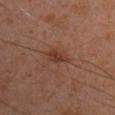Assessment: This lesion was catalogued during total-body skin photography and was not selected for biopsy. Context: Cropped from a whole-body photographic skin survey; the tile spans about 15 mm. A male subject, about 45 years old. On the right upper arm. Captured under cross-polarized illumination. Measured at roughly 3 mm in maximum diameter.